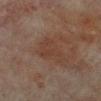Recorded during total-body skin imaging; not selected for excision or biopsy.
Longest diameter approximately 4 mm.
The patient is a female in their 80s.
The total-body-photography lesion software estimated a lesion area of about 8 mm² and a shape-asymmetry score of about 0.55 (0 = symmetric). The software also gave an automated nevus-likeness rating near 0 out of 100.
The lesion is located on the left lower leg.
A region of skin cropped from a whole-body photographic capture, roughly 15 mm wide.